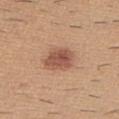biopsy status: no biopsy performed (imaged during a skin exam) | TBP lesion metrics: a lesion color around L≈53 a*≈23 b*≈30 in CIELAB, a lesion–skin lightness drop of about 12, and a lesion-to-skin contrast of about 8.5 (normalized; higher = more distinct); a border-irregularity index near 2/10, a color-variation rating of about 3.5/10, and peripheral color asymmetry of about 1 | diameter: ≈3.5 mm | image: total-body-photography crop, ~15 mm field of view | patient: male, about 60 years old | body site: the upper back | tile lighting: white-light.